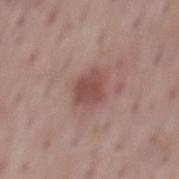  biopsy_status: not biopsied; imaged during a skin examination
  patient:
    sex: male
    age_approx: 55
  lesion_size:
    long_diameter_mm_approx: 3.5
  lighting: white-light
  image:
    source: total-body photography crop
    field_of_view_mm: 15
  site: mid back